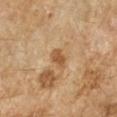Impression: The lesion was tiled from a total-body skin photograph and was not biopsied. Image and clinical context: On the right forearm. Captured under cross-polarized illumination. The total-body-photography lesion software estimated a footprint of about 4 mm² and an eccentricity of roughly 0.8. And it measured a lesion color around L≈55 a*≈21 b*≈39 in CIELAB, roughly 11 lightness units darker than nearby skin, and a lesion-to-skin contrast of about 7.5 (normalized; higher = more distinct). It also reported a border-irregularity rating of about 2.5/10. And it measured a nevus-likeness score of about 0/100. A 15 mm crop from a total-body photograph taken for skin-cancer surveillance. The lesion's longest dimension is about 3 mm. A female patient aged 68–72.